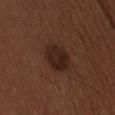The tile uses white-light illumination. Located on the chest. The patient is a male approximately 30 years of age. The lesion's longest dimension is about 3.5 mm. A 15 mm crop from a total-body photograph taken for skin-cancer surveillance.